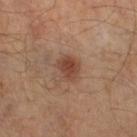Imaged during a routine full-body skin examination; the lesion was not biopsied and no histopathology is available.
Approximately 3 mm at its widest.
Captured under cross-polarized illumination.
Located on the right lower leg.
A 15 mm close-up extracted from a 3D total-body photography capture.
A male patient, aged 53 to 57.